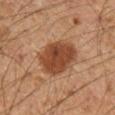Clinical impression: Captured during whole-body skin photography for melanoma surveillance; the lesion was not biopsied.